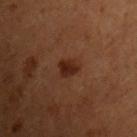acquisition: ~15 mm tile from a whole-body skin photo
subject: male, aged approximately 50
location: the chest
automated metrics: an average lesion color of about L≈19 a*≈18 b*≈22 (CIELAB), a lesion–skin lightness drop of about 8, and a lesion-to-skin contrast of about 9.5 (normalized; higher = more distinct); border irregularity of about 2 on a 0–10 scale and a peripheral color-asymmetry measure near 1; an automated nevus-likeness rating near 95 out of 100 and a lesion-detection confidence of about 100/100
size: about 2.5 mm
tile lighting: cross-polarized